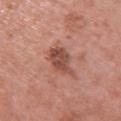notes = catalogued during a skin exam; not biopsied
subject = female, aged approximately 60
illumination = white-light
anatomic site = the back
image = ~15 mm tile from a whole-body skin photo